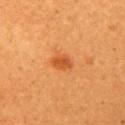Imaged during a routine full-body skin examination; the lesion was not biopsied and no histopathology is available. An algorithmic analysis of the crop reported an area of roughly 4 mm², an eccentricity of roughly 0.65, and a symmetry-axis asymmetry near 0.2. It also reported an average lesion color of about L≈51 a*≈31 b*≈44 (CIELAB), about 10 CIELAB-L* units darker than the surrounding skin, and a normalized border contrast of about 7. The analysis additionally found an automated nevus-likeness rating near 95 out of 100. The tile uses cross-polarized illumination. On the left upper arm. About 2.5 mm across. A female patient aged 28 to 32. A 15 mm close-up extracted from a 3D total-body photography capture.